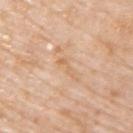Impression:
No biopsy was performed on this lesion — it was imaged during a full skin examination and was not determined to be concerning.
Image and clinical context:
A lesion tile, about 15 mm wide, cut from a 3D total-body photograph. Located on the right upper arm. The lesion-visualizer software estimated a footprint of about 2.5 mm². It also reported a mean CIELAB color near L≈66 a*≈19 b*≈37, roughly 6 lightness units darker than nearby skin, and a lesion-to-skin contrast of about 5.5 (normalized; higher = more distinct). It also reported a border-irregularity index near 7/10, a within-lesion color-variation index near 0/10, and a peripheral color-asymmetry measure near 0. And it measured an automated nevus-likeness rating near 0 out of 100 and a lesion-detection confidence of about 100/100. A male patient aged approximately 75.